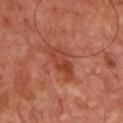biopsy status — no biopsy performed (imaged during a skin exam)
lighting — cross-polarized illumination
lesion size — ~5 mm (longest diameter)
patient — male, about 65 years old
image source — ~15 mm tile from a whole-body skin photo
image-analysis metrics — an area of roughly 8.5 mm², an outline eccentricity of about 0.9 (0 = round, 1 = elongated), and two-axis asymmetry of about 0.35; roughly 8 lightness units darker than nearby skin and a lesion-to-skin contrast of about 6.5 (normalized; higher = more distinct)